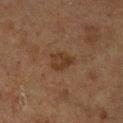lesion_size:
  long_diameter_mm_approx: 3.0
patient:
  sex: male
  age_approx: 75
image:
  source: total-body photography crop
  field_of_view_mm: 15
site: leg
lighting: cross-polarized